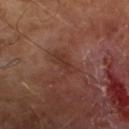– patient · male, roughly 65 years of age
– tile lighting · cross-polarized illumination
– TBP lesion metrics · a footprint of about 5 mm², an eccentricity of roughly 0.9, and two-axis asymmetry of about 0.4
– image · 15 mm crop, total-body photography
– lesion size · about 4 mm